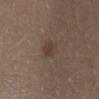Q: Was this lesion biopsied?
A: total-body-photography surveillance lesion; no biopsy
Q: Patient demographics?
A: female, aged 28 to 32
Q: What kind of image is this?
A: ~15 mm crop, total-body skin-cancer survey
Q: Where on the body is the lesion?
A: the abdomen
Q: What lighting was used for the tile?
A: white-light illumination
Q: How large is the lesion?
A: about 2.5 mm